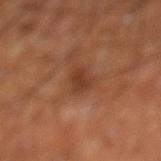notes: imaged on a skin check; not biopsied
illumination: cross-polarized
lesion size: ≈3 mm
image: ~15 mm crop, total-body skin-cancer survey
location: the left lower leg
subject: male, about 60 years old